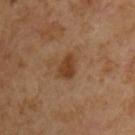The lesion was photographed on a routine skin check and not biopsied; there is no pathology result.
Captured under cross-polarized illumination.
A male patient, aged approximately 65.
Measured at roughly 2.5 mm in maximum diameter.
This image is a 15 mm lesion crop taken from a total-body photograph.